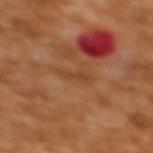{
  "biopsy_status": "not biopsied; imaged during a skin examination",
  "patient": {
    "sex": "female",
    "age_approx": 55
  },
  "lesion_size": {
    "long_diameter_mm_approx": 3.0
  },
  "image": {
    "source": "total-body photography crop",
    "field_of_view_mm": 15
  },
  "site": "upper back",
  "automated_metrics": {
    "eccentricity": 0.95,
    "shape_asymmetry": 0.45,
    "border_irregularity_0_10": 5.0,
    "color_variation_0_10": 0.0,
    "peripheral_color_asymmetry": 0.0
  },
  "lighting": "cross-polarized"
}